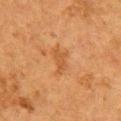| feature | finding |
|---|---|
| subject | female, about 50 years old |
| imaging modality | total-body-photography crop, ~15 mm field of view |
| lighting | cross-polarized illumination |
| body site | the front of the torso |
| lesion diameter | about 3.5 mm |